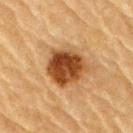Assessment: Recorded during total-body skin imaging; not selected for excision or biopsy. Context: A male patient, approximately 85 years of age or older. Located on the right upper arm. Cropped from a whole-body photographic skin survey; the tile spans about 15 mm.